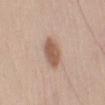workup — imaged on a skin check; not biopsied | illumination — white-light illumination | image — total-body-photography crop, ~15 mm field of view | automated metrics — an outline eccentricity of about 0.7 (0 = round, 1 = elongated); a lesion color around L≈57 a*≈19 b*≈28 in CIELAB and a normalized lesion–skin contrast near 8; border irregularity of about 2 on a 0–10 scale and peripheral color asymmetry of about 1; a lesion-detection confidence of about 100/100 | subject — male, in their mid- to late 40s | site — the left upper arm.